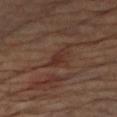Clinical summary:
A roughly 15 mm field-of-view crop from a total-body skin photograph. The total-body-photography lesion software estimated a footprint of about 4 mm², an eccentricity of roughly 0.85, and a symmetry-axis asymmetry near 0.35. And it measured a nevus-likeness score of about 0/100 and a detector confidence of about 90 out of 100 that the crop contains a lesion. About 3 mm across. A male patient, approximately 65 years of age. On the right upper arm.